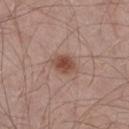Recorded during total-body skin imaging; not selected for excision or biopsy.